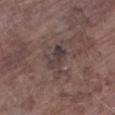<lesion>
<biopsy_status>not biopsied; imaged during a skin examination</biopsy_status>
<image>
  <source>total-body photography crop</source>
  <field_of_view_mm>15</field_of_view_mm>
</image>
<site>left lower leg</site>
<automated_metrics>
  <cielab_L>37</cielab_L>
  <cielab_a>13</cielab_a>
  <cielab_b>15</cielab_b>
  <vs_skin_darker_L>8.0</vs_skin_darker_L>
  <vs_skin_contrast_norm>7.5</vs_skin_contrast_norm>
</automated_metrics>
<patient>
  <sex>male</sex>
  <age_approx>75</age_approx>
</patient>
<lighting>white-light</lighting>
<lesion_size>
  <long_diameter_mm_approx>3.0</long_diameter_mm_approx>
</lesion_size>
</lesion>